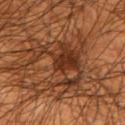Case summary:
- biopsy status: no biopsy performed (imaged during a skin exam)
- tile lighting: cross-polarized illumination
- acquisition: ~15 mm tile from a whole-body skin photo
- site: the left upper arm
- subject: male, approximately 45 years of age